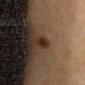No biopsy was performed on this lesion — it was imaged during a full skin examination and was not determined to be concerning. The lesion is on the chest. A female patient, aged 28 to 32. A 15 mm crop from a total-body photograph taken for skin-cancer surveillance. The lesion-visualizer software estimated a shape eccentricity near 0.8 and a shape-asymmetry score of about 0.35 (0 = symmetric). And it measured a border-irregularity index near 2.5/10, internal color variation of about 0 on a 0–10 scale, and radial color variation of about 0. The software also gave an automated nevus-likeness rating near 100 out of 100 and lesion-presence confidence of about 100/100.